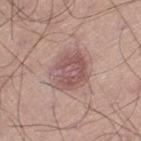Part of a total-body skin-imaging series; this lesion was reviewed on a skin check and was not flagged for biopsy.
This is a white-light tile.
Automated image analysis of the tile measured an area of roughly 15 mm² and an outline eccentricity of about 0.55 (0 = round, 1 = elongated). And it measured an automated nevus-likeness rating near 20 out of 100 and lesion-presence confidence of about 100/100.
Approximately 5 mm at its widest.
On the left thigh.
This image is a 15 mm lesion crop taken from a total-body photograph.
A male subject, about 45 years old.